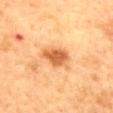follow-up — imaged on a skin check; not biopsied | lesion size — ≈3.5 mm | acquisition — 15 mm crop, total-body photography | automated metrics — an area of roughly 7 mm² and an outline eccentricity of about 0.7 (0 = round, 1 = elongated); a lesion color around L≈56 a*≈25 b*≈41 in CIELAB, a lesion–skin lightness drop of about 14, and a normalized lesion–skin contrast near 9; a nevus-likeness score of about 95/100 and a lesion-detection confidence of about 100/100 | anatomic site — the mid back | subject — female, aged approximately 60.